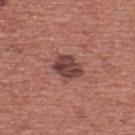Captured during whole-body skin photography for melanoma surveillance; the lesion was not biopsied.
Longest diameter approximately 3.5 mm.
A 15 mm close-up extracted from a 3D total-body photography capture.
Imaged with white-light lighting.
A male patient approximately 45 years of age.
From the chest.